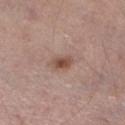notes = imaged on a skin check; not biopsied | acquisition = ~15 mm tile from a whole-body skin photo | lighting = white-light | patient = male, aged approximately 55 | lesion size = ~3 mm (longest diameter) | location = the right lower leg.